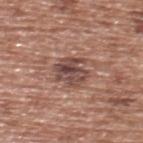Clinical impression:
Captured during whole-body skin photography for melanoma surveillance; the lesion was not biopsied.
Image and clinical context:
A 15 mm crop from a total-body photograph taken for skin-cancer surveillance. Located on the upper back. The lesion's longest dimension is about 3.5 mm. An algorithmic analysis of the crop reported a footprint of about 9 mm², a shape eccentricity near 0.4, and a shape-asymmetry score of about 0.3 (0 = symmetric). A male subject in their mid- to late 70s.